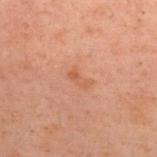Findings:
* workup · imaged on a skin check; not biopsied
* tile lighting · cross-polarized illumination
* patient · male, in their 40s
* TBP lesion metrics · a lesion color around L≈46 a*≈21 b*≈29 in CIELAB, roughly 5 lightness units darker than nearby skin, and a normalized border contrast of about 5; a color-variation rating of about 0.5/10
* image · 15 mm crop, total-body photography
* location · the upper back
* diameter · ~3 mm (longest diameter)